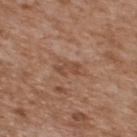Notes:
• follow-up: no biopsy performed (imaged during a skin exam)
• site: the upper back
• patient: male, in their mid- to late 60s
• image: ~15 mm crop, total-body skin-cancer survey